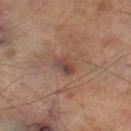Recorded during total-body skin imaging; not selected for excision or biopsy. Measured at roughly 3 mm in maximum diameter. The tile uses cross-polarized illumination. On the left thigh. Cropped from a whole-body photographic skin survey; the tile spans about 15 mm. An algorithmic analysis of the crop reported a footprint of about 4 mm² and a symmetry-axis asymmetry near 0.25. The software also gave an average lesion color of about L≈43 a*≈19 b*≈23 (CIELAB), roughly 8 lightness units darker than nearby skin, and a lesion-to-skin contrast of about 7 (normalized; higher = more distinct). And it measured a border-irregularity rating of about 2.5/10, internal color variation of about 4.5 on a 0–10 scale, and peripheral color asymmetry of about 1.5. It also reported a nevus-likeness score of about 0/100 and a detector confidence of about 100 out of 100 that the crop contains a lesion. A male subject approximately 70 years of age.